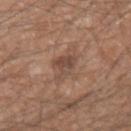{"biopsy_status": "not biopsied; imaged during a skin examination", "lighting": "white-light", "patient": {"sex": "male", "age_approx": 50}, "automated_metrics": {"area_mm2_approx": 6.5, "shape_asymmetry": 0.35, "cielab_L": 47, "cielab_a": 18, "cielab_b": 26, "vs_skin_darker_L": 9.0, "vs_skin_contrast_norm": 7.0}, "lesion_size": {"long_diameter_mm_approx": 4.0}, "image": {"source": "total-body photography crop", "field_of_view_mm": 15}, "site": "left forearm"}